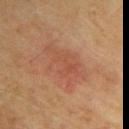The lesion was photographed on a routine skin check and not biopsied; there is no pathology result.